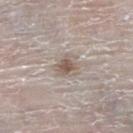Imaged during a routine full-body skin examination; the lesion was not biopsied and no histopathology is available. The patient is a male in their 80s. On the right lower leg. A 15 mm close-up tile from a total-body photography series done for melanoma screening.